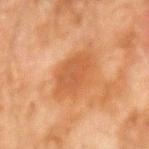No biopsy was performed on this lesion — it was imaged during a full skin examination and was not determined to be concerning.
A male subject aged 43 to 47.
About 5 mm across.
Located on the right forearm.
An algorithmic analysis of the crop reported a nevus-likeness score of about 55/100 and lesion-presence confidence of about 100/100.
Cropped from a whole-body photographic skin survey; the tile spans about 15 mm.